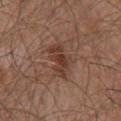The lesion was photographed on a routine skin check and not biopsied; there is no pathology result. The total-body-photography lesion software estimated a normalized lesion–skin contrast near 7.5. The software also gave a border-irregularity rating of about 3.5/10, internal color variation of about 3.5 on a 0–10 scale, and a peripheral color-asymmetry measure near 1. The tile uses white-light illumination. Located on the chest. The subject is a male in their mid- to late 60s. Cropped from a whole-body photographic skin survey; the tile spans about 15 mm. Approximately 4.5 mm at its widest.